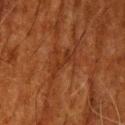The lesion was photographed on a routine skin check and not biopsied; there is no pathology result.
A 15 mm close-up tile from a total-body photography series done for melanoma screening.
The subject is a male aged 78–82.
An algorithmic analysis of the crop reported a lesion area of about 4.5 mm², an outline eccentricity of about 0.95 (0 = round, 1 = elongated), and a symmetry-axis asymmetry near 0.45. It also reported a classifier nevus-likeness of about 0/100 and a detector confidence of about 65 out of 100 that the crop contains a lesion.
The tile uses cross-polarized illumination.
Located on the head or neck.